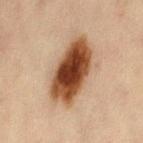Captured under cross-polarized illumination. A 15 mm crop from a total-body photograph taken for skin-cancer surveillance. The lesion-visualizer software estimated an average lesion color of about L≈39 a*≈21 b*≈31 (CIELAB) and about 21 CIELAB-L* units darker than the surrounding skin. The analysis additionally found border irregularity of about 2 on a 0–10 scale, a within-lesion color-variation index near 7/10, and radial color variation of about 2. The software also gave an automated nevus-likeness rating near 100 out of 100 and a lesion-detection confidence of about 100/100. About 7.5 mm across. The lesion is on the left thigh. A female subject aged approximately 45.